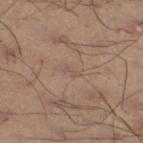{
  "biopsy_status": "not biopsied; imaged during a skin examination",
  "lesion_size": {
    "long_diameter_mm_approx": 2.0
  },
  "patient": {
    "sex": "male",
    "age_approx": 55
  },
  "site": "left lower leg",
  "lighting": "white-light",
  "image": {
    "source": "total-body photography crop",
    "field_of_view_mm": 15
  }
}